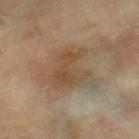notes — imaged on a skin check; not biopsied
image source — ~15 mm tile from a whole-body skin photo
patient — in their 60s
anatomic site — the right lower leg